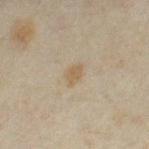Findings:
– notes — imaged on a skin check; not biopsied
– site — the left thigh
– image source — 15 mm crop, total-body photography
– subject — female, aged 33 to 37